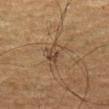Part of a total-body skin-imaging series; this lesion was reviewed on a skin check and was not flagged for biopsy. Imaged with cross-polarized lighting. A male subject in their mid- to late 70s. On the left thigh. Cropped from a whole-body photographic skin survey; the tile spans about 15 mm.